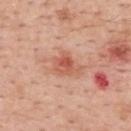<record>
  <biopsy_status>not biopsied; imaged during a skin examination</biopsy_status>
  <site>upper back</site>
  <image>
    <source>total-body photography crop</source>
    <field_of_view_mm>15</field_of_view_mm>
  </image>
  <patient>
    <sex>female</sex>
    <age_approx>50</age_approx>
  </patient>
  <lighting>white-light</lighting>
  <automated_metrics>
    <color_variation_0_10>5.5</color_variation_0_10>
  </automated_metrics>
</record>